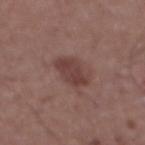{
  "biopsy_status": "not biopsied; imaged during a skin examination",
  "lighting": "white-light",
  "patient": {
    "sex": "male",
    "age_approx": 55
  },
  "image": {
    "source": "total-body photography crop",
    "field_of_view_mm": 15
  },
  "site": "front of the torso"
}